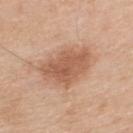biopsy status: total-body-photography surveillance lesion; no biopsy | automated lesion analysis: a lesion color around L≈58 a*≈22 b*≈33 in CIELAB and roughly 11 lightness units darker than nearby skin; a nevus-likeness score of about 85/100 and a detector confidence of about 100 out of 100 that the crop contains a lesion | lesion size: ≈6 mm | anatomic site: the upper back | image source: total-body-photography crop, ~15 mm field of view | subject: male, roughly 60 years of age | lighting: white-light illumination.